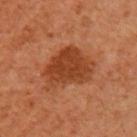Notes:
• biopsy status: imaged on a skin check; not biopsied
• lesion diameter: ≈6 mm
• patient: male, roughly 45 years of age
• image source: total-body-photography crop, ~15 mm field of view
• site: the arm